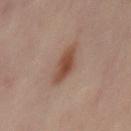Q: Was this lesion biopsied?
A: total-body-photography surveillance lesion; no biopsy
Q: What are the patient's age and sex?
A: female, roughly 50 years of age
Q: How was this image acquired?
A: 15 mm crop, total-body photography
Q: What is the anatomic site?
A: the front of the torso